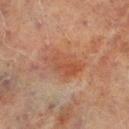{
  "biopsy_status": "not biopsied; imaged during a skin examination",
  "site": "right lower leg",
  "image": {
    "source": "total-body photography crop",
    "field_of_view_mm": 15
  },
  "lighting": "cross-polarized",
  "automated_metrics": {
    "area_mm2_approx": 10.0,
    "eccentricity": 0.8,
    "shape_asymmetry": 0.35,
    "vs_skin_darker_L": 6.0,
    "vs_skin_contrast_norm": 6.0,
    "lesion_detection_confidence_0_100": 100
  },
  "lesion_size": {
    "long_diameter_mm_approx": 5.0
  },
  "patient": {
    "sex": "male",
    "age_approx": 70
  }
}